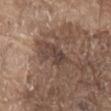A close-up tile cropped from a whole-body skin photograph, about 15 mm across. The patient is a male approximately 80 years of age. This is a white-light tile. The lesion is on the chest. The lesion's longest dimension is about 4 mm.